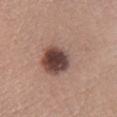Assessment:
Imaged during a routine full-body skin examination; the lesion was not biopsied and no histopathology is available.
Context:
From the left lower leg. A 15 mm crop from a total-body photograph taken for skin-cancer surveillance. Imaged with white-light lighting. Longest diameter approximately 7.5 mm. A male patient roughly 40 years of age.